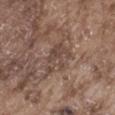lighting = white-light illumination; location = the left thigh; imaging modality = total-body-photography crop, ~15 mm field of view; patient = male, aged 73–77; lesion size = ≈4 mm.